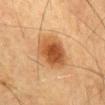Impression: Recorded during total-body skin imaging; not selected for excision or biopsy. Context: The lesion-visualizer software estimated an average lesion color of about L≈47 a*≈22 b*≈37 (CIELAB). The software also gave a classifier nevus-likeness of about 100/100 and a detector confidence of about 100 out of 100 that the crop contains a lesion. Located on the front of the torso. A male patient, aged 83 or older. A 15 mm close-up extracted from a 3D total-body photography capture.